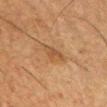No biopsy was performed on this lesion — it was imaged during a full skin examination and was not determined to be concerning.
The tile uses cross-polarized illumination.
A male patient, about 50 years old.
On the chest.
A close-up tile cropped from a whole-body skin photograph, about 15 mm across.